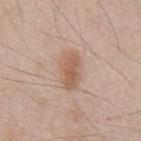Captured during whole-body skin photography for melanoma surveillance; the lesion was not biopsied. Captured under white-light illumination. On the abdomen. Measured at roughly 4.5 mm in maximum diameter. Cropped from a whole-body photographic skin survey; the tile spans about 15 mm. The patient is a male aged approximately 45.